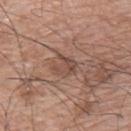Impression:
Part of a total-body skin-imaging series; this lesion was reviewed on a skin check and was not flagged for biopsy.
Context:
A 15 mm close-up tile from a total-body photography series done for melanoma screening. A male patient aged 63–67. The tile uses white-light illumination. The lesion is on the upper back.